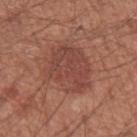Q: Is there a histopathology result?
A: catalogued during a skin exam; not biopsied
Q: What kind of image is this?
A: total-body-photography crop, ~15 mm field of view
Q: What is the lesion's diameter?
A: ≈6 mm
Q: Patient demographics?
A: male, aged 58–62
Q: What is the anatomic site?
A: the left forearm
Q: What lighting was used for the tile?
A: white-light illumination